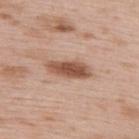Recorded during total-body skin imaging; not selected for excision or biopsy. A male patient, aged 48–52. The lesion's longest dimension is about 5 mm. Captured under white-light illumination. On the upper back. A 15 mm close-up extracted from a 3D total-body photography capture.